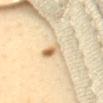workup: no biopsy performed (imaged during a skin exam) | TBP lesion metrics: an area of roughly 3.5 mm² and a shape eccentricity near 0.85; internal color variation of about 7 on a 0–10 scale and peripheral color asymmetry of about 3; a classifier nevus-likeness of about 100/100 and lesion-presence confidence of about 100/100 | lighting: cross-polarized | subject: female, roughly 55 years of age | body site: the mid back | image source: ~15 mm tile from a whole-body skin photo | diameter: ~2.5 mm (longest diameter).